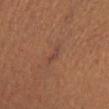{"biopsy_status": "not biopsied; imaged during a skin examination", "patient": {"sex": "female", "age_approx": 65}, "lighting": "cross-polarized", "lesion_size": {"long_diameter_mm_approx": 2.5}, "site": "left lower leg", "automated_metrics": {"area_mm2_approx": 2.0, "eccentricity": 0.95, "shape_asymmetry": 0.55}, "image": {"source": "total-body photography crop", "field_of_view_mm": 15}}